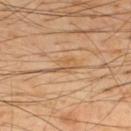Q: Was this lesion biopsied?
A: total-body-photography surveillance lesion; no biopsy
Q: What is the imaging modality?
A: 15 mm crop, total-body photography
Q: Who is the patient?
A: male, aged around 50
Q: Where on the body is the lesion?
A: the upper back